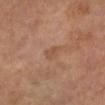Impression: Imaged during a routine full-body skin examination; the lesion was not biopsied and no histopathology is available. Background: Measured at roughly 2.5 mm in maximum diameter. The subject is a male approximately 65 years of age. A close-up tile cropped from a whole-body skin photograph, about 15 mm across. The tile uses cross-polarized illumination. Automated tile analysis of the lesion measured a symmetry-axis asymmetry near 0.4. The analysis additionally found an average lesion color of about L≈48 a*≈20 b*≈30 (CIELAB), a lesion–skin lightness drop of about 6, and a lesion-to-skin contrast of about 5 (normalized; higher = more distinct). The software also gave a lesion-detection confidence of about 100/100. The lesion is on the leg.